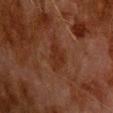notes — total-body-photography surveillance lesion; no biopsy | illumination — cross-polarized | imaging modality — ~15 mm crop, total-body skin-cancer survey | patient — male, in their 80s | size — about 4 mm | image-analysis metrics — an average lesion color of about L≈23 a*≈19 b*≈24 (CIELAB), about 4 CIELAB-L* units darker than the surrounding skin, and a normalized border contrast of about 6; a nevus-likeness score of about 5/100 | location — the upper back.